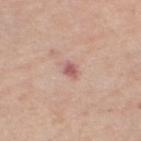Clinical impression: Imaged during a routine full-body skin examination; the lesion was not biopsied and no histopathology is available. Acquisition and patient details: The recorded lesion diameter is about 2.5 mm. The lesion is located on the left thigh. An algorithmic analysis of the crop reported a lesion color around L≈58 a*≈24 b*≈22 in CIELAB and a normalized border contrast of about 7.5. The software also gave a detector confidence of about 100 out of 100 that the crop contains a lesion. A 15 mm crop from a total-body photograph taken for skin-cancer surveillance. A female subject, aged 63–67. Imaged with white-light lighting.